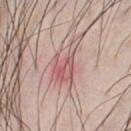biopsy status: imaged on a skin check; not biopsied | body site: the chest | patient: male, aged 33–37 | lighting: white-light | automated lesion analysis: a border-irregularity rating of about 8.5/10, a within-lesion color-variation index near 5.5/10, and radial color variation of about 2; an automated nevus-likeness rating near 0 out of 100 and lesion-presence confidence of about 95/100 | imaging modality: 15 mm crop, total-body photography.